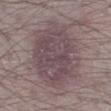Q: Who is the patient?
A: male, approximately 50 years of age
Q: Automated lesion metrics?
A: a lesion area of about 42 mm², an outline eccentricity of about 0.65 (0 = round, 1 = elongated), and a symmetry-axis asymmetry near 0.15
Q: What kind of image is this?
A: ~15 mm tile from a whole-body skin photo
Q: What is the lesion's diameter?
A: ≈8.5 mm
Q: What is the anatomic site?
A: the right lower leg
Q: How was the tile lit?
A: white-light illumination